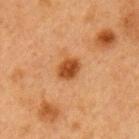anatomic site: the left upper arm | illumination: cross-polarized illumination | TBP lesion metrics: an average lesion color of about L≈42 a*≈25 b*≈38 (CIELAB), a lesion–skin lightness drop of about 13, and a lesion-to-skin contrast of about 10.5 (normalized; higher = more distinct); border irregularity of about 1.5 on a 0–10 scale, a within-lesion color-variation index near 3/10, and radial color variation of about 1 | acquisition: 15 mm crop, total-body photography | patient: female, approximately 40 years of age | lesion diameter: ~3 mm (longest diameter).